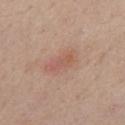The subject is a male aged 53–57.
This image is a 15 mm lesion crop taken from a total-body photograph.
The lesion is on the chest.